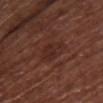The lesion was photographed on a routine skin check and not biopsied; there is no pathology result.
The lesion is on the chest.
A lesion tile, about 15 mm wide, cut from a 3D total-body photograph.
A female subject, in their 80s.
This is a white-light tile.
Measured at roughly 3 mm in maximum diameter.